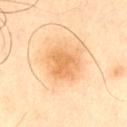This lesion was catalogued during total-body skin photography and was not selected for biopsy.
The lesion is located on the left upper arm.
Captured under cross-polarized illumination.
A lesion tile, about 15 mm wide, cut from a 3D total-body photograph.
A male patient in their mid-60s.
Measured at roughly 3.5 mm in maximum diameter.